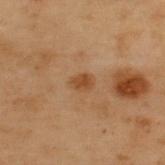biopsy status=total-body-photography surveillance lesion; no biopsy
anatomic site=the upper back
patient=male, in their mid- to late 50s
imaging modality=total-body-photography crop, ~15 mm field of view